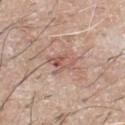This lesion was catalogued during total-body skin photography and was not selected for biopsy.
The total-body-photography lesion software estimated a mean CIELAB color near L≈57 a*≈20 b*≈25.
The tile uses white-light illumination.
A male patient, in their mid- to late 60s.
From the chest.
Approximately 3.5 mm at its widest.
A 15 mm close-up extracted from a 3D total-body photography capture.